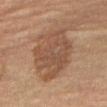<record>
<biopsy_status>not biopsied; imaged during a skin examination</biopsy_status>
<automated_metrics>
  <area_mm2_approx>27.0</area_mm2_approx>
  <shape_asymmetry>0.25</shape_asymmetry>
  <cielab_L>48</cielab_L>
  <cielab_a>18</cielab_a>
  <cielab_b>29</cielab_b>
  <vs_skin_darker_L>9.0</vs_skin_darker_L>
  <vs_skin_contrast_norm>6.5</vs_skin_contrast_norm>
</automated_metrics>
<lesion_size>
  <long_diameter_mm_approx>6.5</long_diameter_mm_approx>
</lesion_size>
<image>
  <source>total-body photography crop</source>
  <field_of_view_mm>15</field_of_view_mm>
</image>
<site>right thigh</site>
<patient>
  <sex>female</sex>
  <age_approx>70</age_approx>
</patient>
</record>